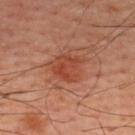Background:
Approximately 4 mm at its widest. A roughly 15 mm field-of-view crop from a total-body skin photograph. The tile uses cross-polarized illumination. The lesion is located on the upper back. A male subject, approximately 60 years of age.